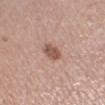notes: catalogued during a skin exam; not biopsied
lesion size: ~3 mm (longest diameter)
patient: female, aged 63 to 67
imaging modality: total-body-photography crop, ~15 mm field of view
site: the left lower leg
image-analysis metrics: a classifier nevus-likeness of about 50/100 and a lesion-detection confidence of about 100/100
illumination: white-light